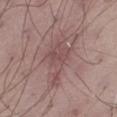* biopsy status · no biopsy performed (imaged during a skin exam)